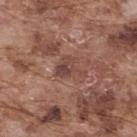The lesion was photographed on a routine skin check and not biopsied; there is no pathology result.
The lesion is on the upper back.
The lesion's longest dimension is about 3.5 mm.
Automated tile analysis of the lesion measured an area of roughly 5 mm² and a shape eccentricity near 0.6. The software also gave a border-irregularity rating of about 5.5/10, internal color variation of about 4.5 on a 0–10 scale, and a peripheral color-asymmetry measure near 1.5. The analysis additionally found a nevus-likeness score of about 0/100 and a detector confidence of about 95 out of 100 that the crop contains a lesion.
Captured under white-light illumination.
A male patient, in their mid- to late 70s.
A 15 mm close-up extracted from a 3D total-body photography capture.